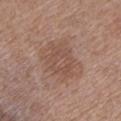Clinical impression: Imaged during a routine full-body skin examination; the lesion was not biopsied and no histopathology is available. Context: About 5.5 mm across. This image is a 15 mm lesion crop taken from a total-body photograph. Captured under white-light illumination. On the left lower leg. The patient is a female aged around 55.